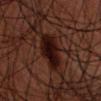{"biopsy_status": "not biopsied; imaged during a skin examination"}